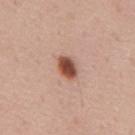* biopsy status: total-body-photography surveillance lesion; no biopsy
* tile lighting: white-light illumination
* imaging modality: ~15 mm crop, total-body skin-cancer survey
* location: the mid back
* lesion size: ~3.5 mm (longest diameter)
* subject: male, roughly 55 years of age